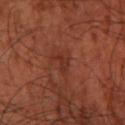Recorded during total-body skin imaging; not selected for excision or biopsy.
About 2.5 mm across.
A patient aged 63–67.
The lesion is located on the left thigh.
The lesion-visualizer software estimated a lesion color around L≈32 a*≈26 b*≈28 in CIELAB and roughly 6 lightness units darker than nearby skin. And it measured a border-irregularity index near 5.5/10, a within-lesion color-variation index near 0/10, and radial color variation of about 0. The analysis additionally found an automated nevus-likeness rating near 0 out of 100 and lesion-presence confidence of about 95/100.
A close-up tile cropped from a whole-body skin photograph, about 15 mm across.
Captured under cross-polarized illumination.